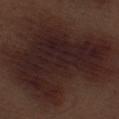The lesion was photographed on a routine skin check and not biopsied; there is no pathology result.
On the left thigh.
The tile uses white-light illumination.
A male subject aged 68–72.
A 15 mm close-up extracted from a 3D total-body photography capture.